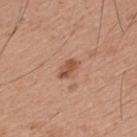{
  "biopsy_status": "not biopsied; imaged during a skin examination",
  "site": "upper back",
  "patient": {
    "sex": "male",
    "age_approx": 30
  },
  "image": {
    "source": "total-body photography crop",
    "field_of_view_mm": 15
  }
}